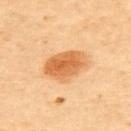biopsy status = catalogued during a skin exam; not biopsied | image source = total-body-photography crop, ~15 mm field of view | anatomic site = the upper back | illumination = cross-polarized illumination | TBP lesion metrics = a lesion area of about 14 mm² and a symmetry-axis asymmetry near 0.15 | subject = male, aged approximately 65.